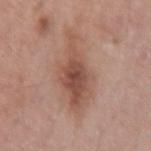This lesion was catalogued during total-body skin photography and was not selected for biopsy. A female patient, in their 40s. Cropped from a total-body skin-imaging series; the visible field is about 15 mm. Located on the right forearm. This is a white-light tile.